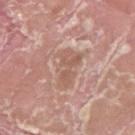• follow-up · no biopsy performed (imaged during a skin exam)
• location · the right thigh
• TBP lesion metrics · a lesion area of about 10 mm² and an outline eccentricity of about 0.9 (0 = round, 1 = elongated); an average lesion color of about L≈58 a*≈20 b*≈26 (CIELAB), a lesion–skin lightness drop of about 7, and a normalized lesion–skin contrast near 5; a border-irregularity index near 5/10, a color-variation rating of about 3/10, and radial color variation of about 1; a classifier nevus-likeness of about 0/100
• lesion size · ~5 mm (longest diameter)
• patient · male, aged around 40
• imaging modality · 15 mm crop, total-body photography